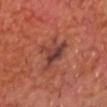Impression:
This lesion was catalogued during total-body skin photography and was not selected for biopsy.
Background:
Measured at roughly 5 mm in maximum diameter. A male patient, aged 63–67. Cropped from a total-body skin-imaging series; the visible field is about 15 mm. The tile uses cross-polarized illumination.